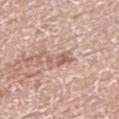<lesion>
  <biopsy_status>not biopsied; imaged during a skin examination</biopsy_status>
  <lesion_size>
    <long_diameter_mm_approx>3.5</long_diameter_mm_approx>
  </lesion_size>
  <automated_metrics>
    <eccentricity>0.85</eccentricity>
    <shape_asymmetry>0.3</shape_asymmetry>
    <border_irregularity_0_10>3.5</border_irregularity_0_10>
    <color_variation_0_10>4.5</color_variation_0_10>
    <peripheral_color_asymmetry>2.0</peripheral_color_asymmetry>
  </automated_metrics>
  <lighting>white-light</lighting>
  <image>
    <source>total-body photography crop</source>
    <field_of_view_mm>15</field_of_view_mm>
  </image>
  <patient>
    <sex>male</sex>
    <age_approx>75</age_approx>
  </patient>
  <site>right thigh</site>
</lesion>